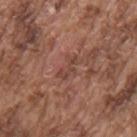| key | value |
|---|---|
| TBP lesion metrics | a lesion area of about 3.5 mm², an outline eccentricity of about 0.9 (0 = round, 1 = elongated), and a symmetry-axis asymmetry near 0.5; roughly 7 lightness units darker than nearby skin and a lesion-to-skin contrast of about 5.5 (normalized; higher = more distinct) |
| anatomic site | the upper back |
| imaging modality | ~15 mm tile from a whole-body skin photo |
| lesion size | ~3 mm (longest diameter) |
| patient | male, in their mid-70s |
| lighting | white-light illumination |